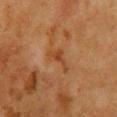Recorded during total-body skin imaging; not selected for excision or biopsy.
A female patient, aged approximately 50.
A 15 mm close-up extracted from a 3D total-body photography capture.
From the front of the torso.
This is a cross-polarized tile.
About 4 mm across.